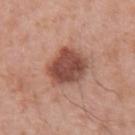The lesion is on the right upper arm. The subject is a female roughly 40 years of age. A lesion tile, about 15 mm wide, cut from a 3D total-body photograph.